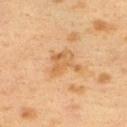workup: total-body-photography surveillance lesion; no biopsy | tile lighting: cross-polarized | image source: ~15 mm crop, total-body skin-cancer survey | patient: female, aged 38–42 | site: the upper back | diameter: ≈4 mm.